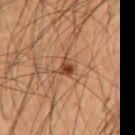Notes:
- workup — no biopsy performed (imaged during a skin exam)
- patient — male, aged approximately 55
- automated lesion analysis — a mean CIELAB color near L≈44 a*≈23 b*≈32, about 10 CIELAB-L* units darker than the surrounding skin, and a normalized border contrast of about 8; border irregularity of about 2.5 on a 0–10 scale and radial color variation of about 3
- location — the chest
- lesion diameter — about 2 mm
- acquisition — ~15 mm crop, total-body skin-cancer survey